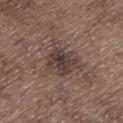biopsy_status: not biopsied; imaged during a skin examination
site: left lower leg
lighting: white-light
automated_metrics:
  area_mm2_approx: 13.0
  eccentricity: 0.5
  shape_asymmetry: 0.35
  border_irregularity_0_10: 4.0
  color_variation_0_10: 4.5
  peripheral_color_asymmetry: 1.5
image:
  source: total-body photography crop
  field_of_view_mm: 15
patient:
  sex: male
  age_approx: 70